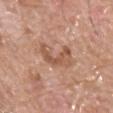Impression:
Captured during whole-body skin photography for melanoma surveillance; the lesion was not biopsied.